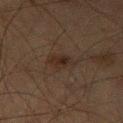Impression:
The lesion was photographed on a routine skin check and not biopsied; there is no pathology result.
Acquisition and patient details:
The lesion is on the right thigh. Captured under cross-polarized illumination. The subject is a male aged approximately 65. Automated tile analysis of the lesion measured an area of roughly 6 mm² and a shape eccentricity near 0.8. It also reported a nevus-likeness score of about 25/100 and a detector confidence of about 100 out of 100 that the crop contains a lesion. The recorded lesion diameter is about 3.5 mm. A close-up tile cropped from a whole-body skin photograph, about 15 mm across.